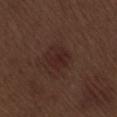A close-up tile cropped from a whole-body skin photograph, about 15 mm across.
A male patient about 70 years old.
Located on the right thigh.